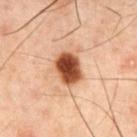follow-up: no biopsy performed (imaged during a skin exam) | acquisition: total-body-photography crop, ~15 mm field of view | patient: male, in their 60s | body site: the chest | lesion diameter: ~3.5 mm (longest diameter).